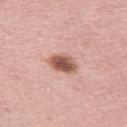Part of a total-body skin-imaging series; this lesion was reviewed on a skin check and was not flagged for biopsy. A female subject, approximately 65 years of age. The lesion's longest dimension is about 3.5 mm. This is a white-light tile. Located on the left thigh. A 15 mm close-up extracted from a 3D total-body photography capture.